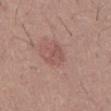Assessment:
This lesion was catalogued during total-body skin photography and was not selected for biopsy.
Context:
This image is a 15 mm lesion crop taken from a total-body photograph. The lesion-visualizer software estimated an area of roughly 4.5 mm², a shape eccentricity near 0.8, and a symmetry-axis asymmetry near 0.4. The analysis additionally found an automated nevus-likeness rating near 5 out of 100 and lesion-presence confidence of about 100/100. Located on the front of the torso. This is a white-light tile. The patient is a male roughly 45 years of age. The lesion's longest dimension is about 3 mm.